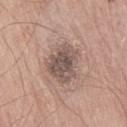Clinical impression: This lesion was catalogued during total-body skin photography and was not selected for biopsy. Acquisition and patient details: The lesion is located on the right thigh. Automated image analysis of the tile measured an average lesion color of about L≈52 a*≈15 b*≈22 (CIELAB) and about 12 CIELAB-L* units darker than the surrounding skin. The software also gave an automated nevus-likeness rating near 0 out of 100 and lesion-presence confidence of about 100/100. The patient is a male roughly 75 years of age. A 15 mm crop from a total-body photograph taken for skin-cancer surveillance. The lesion's longest dimension is about 5 mm.